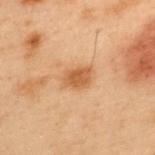Q: Is there a histopathology result?
A: imaged on a skin check; not biopsied
Q: What is the imaging modality?
A: ~15 mm tile from a whole-body skin photo
Q: What is the anatomic site?
A: the back
Q: Lesion size?
A: about 3.5 mm
Q: What are the patient's age and sex?
A: male, aged 53 to 57
Q: What lighting was used for the tile?
A: cross-polarized illumination